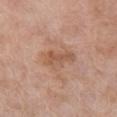notes — no biopsy performed (imaged during a skin exam); imaging modality — ~15 mm crop, total-body skin-cancer survey; lesion diameter — ≈4 mm; tile lighting — white-light illumination; patient — female, roughly 75 years of age; location — the arm.